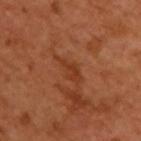Q: Is there a histopathology result?
A: catalogued during a skin exam; not biopsied
Q: What is the anatomic site?
A: the back
Q: What is the imaging modality?
A: ~15 mm crop, total-body skin-cancer survey
Q: How was the tile lit?
A: cross-polarized illumination
Q: Automated lesion metrics?
A: a mean CIELAB color near L≈38 a*≈27 b*≈35 and about 7 CIELAB-L* units darker than the surrounding skin; a nevus-likeness score of about 0/100 and lesion-presence confidence of about 90/100
Q: What are the patient's age and sex?
A: male, aged around 50
Q: Lesion size?
A: ~4 mm (longest diameter)